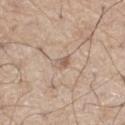Q: Where on the body is the lesion?
A: the right thigh
Q: Automated lesion metrics?
A: border irregularity of about 3 on a 0–10 scale, internal color variation of about 6 on a 0–10 scale, and radial color variation of about 2; a nevus-likeness score of about 0/100
Q: Who is the patient?
A: male, aged around 70
Q: What is the lesion's diameter?
A: ≈2.5 mm
Q: What kind of image is this?
A: 15 mm crop, total-body photography